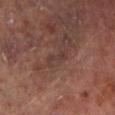• workup · imaged on a skin check; not biopsied
• illumination · cross-polarized illumination
• lesion diameter · ≈3 mm
• body site · the left lower leg
• patient · male, approximately 65 years of age
• automated metrics · an area of roughly 2.5 mm² and a shape eccentricity near 0.95; a color-variation rating of about 0/10; a classifier nevus-likeness of about 0/100 and a lesion-detection confidence of about 80/100
• imaging modality · ~15 mm tile from a whole-body skin photo